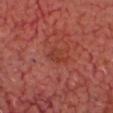Case summary:
– notes — no biopsy performed (imaged during a skin exam)
– body site — the head or neck
– automated lesion analysis — an outline eccentricity of about 0.85 (0 = round, 1 = elongated) and a symmetry-axis asymmetry near 0.5
– patient — aged 63 to 67
– image source — ~15 mm tile from a whole-body skin photo